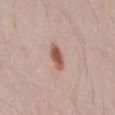Impression:
Imaged during a routine full-body skin examination; the lesion was not biopsied and no histopathology is available.
Image and clinical context:
Automated tile analysis of the lesion measured a lesion area of about 5 mm² and a shape-asymmetry score of about 0.2 (0 = symmetric). The analysis additionally found a mean CIELAB color near L≈56 a*≈22 b*≈28, about 13 CIELAB-L* units darker than the surrounding skin, and a normalized border contrast of about 9.5. The analysis additionally found a border-irregularity rating of about 2/10, a color-variation rating of about 3/10, and peripheral color asymmetry of about 1. On the lower back. Longest diameter approximately 3 mm. Captured under white-light illumination. A male patient, aged 23–27. Cropped from a whole-body photographic skin survey; the tile spans about 15 mm.